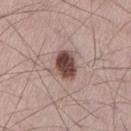Clinical impression: Captured during whole-body skin photography for melanoma surveillance; the lesion was not biopsied. Acquisition and patient details: A close-up tile cropped from a whole-body skin photograph, about 15 mm across. The recorded lesion diameter is about 3.5 mm. A male patient about 70 years old. Automated tile analysis of the lesion measured a lesion area of about 9 mm², an eccentricity of roughly 0.55, and a shape-asymmetry score of about 0.2 (0 = symmetric). The software also gave a lesion color around L≈47 a*≈18 b*≈21 in CIELAB, roughly 17 lightness units darker than nearby skin, and a lesion-to-skin contrast of about 11.5 (normalized; higher = more distinct). And it measured a nevus-likeness score of about 95/100 and a detector confidence of about 100 out of 100 that the crop contains a lesion. The tile uses white-light illumination. The lesion is on the leg.